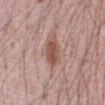The subject is a male aged approximately 70. About 4 mm across. Cropped from a whole-body photographic skin survey; the tile spans about 15 mm. Located on the abdomen. Automated image analysis of the tile measured a classifier nevus-likeness of about 90/100 and a detector confidence of about 100 out of 100 that the crop contains a lesion.